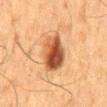| key | value |
|---|---|
| workup | catalogued during a skin exam; not biopsied |
| lesion diameter | ≈5.5 mm |
| location | the mid back |
| tile lighting | cross-polarized illumination |
| patient | male, roughly 60 years of age |
| acquisition | 15 mm crop, total-body photography |